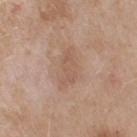Q: Was this lesion biopsied?
A: imaged on a skin check; not biopsied
Q: What is the anatomic site?
A: the right upper arm
Q: Patient demographics?
A: female, about 55 years old
Q: What kind of image is this?
A: ~15 mm tile from a whole-body skin photo